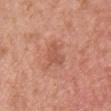Part of a total-body skin-imaging series; this lesion was reviewed on a skin check and was not flagged for biopsy.
The total-body-photography lesion software estimated border irregularity of about 5.5 on a 0–10 scale, a color-variation rating of about 1/10, and radial color variation of about 0.5. The software also gave a classifier nevus-likeness of about 5/100 and lesion-presence confidence of about 100/100.
From the chest.
The subject is a female approximately 40 years of age.
A 15 mm crop from a total-body photograph taken for skin-cancer surveillance.
Captured under white-light illumination.